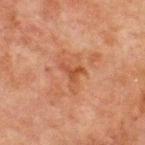biopsy status: imaged on a skin check; not biopsied
lesion size: ~3 mm (longest diameter)
subject: male, approximately 65 years of age
image-analysis metrics: a lesion area of about 3 mm² and a shape-asymmetry score of about 0.7 (0 = symmetric)
body site: the chest
image source: ~15 mm tile from a whole-body skin photo
tile lighting: cross-polarized illumination